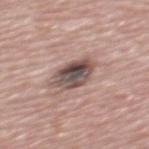Part of a total-body skin-imaging series; this lesion was reviewed on a skin check and was not flagged for biopsy.
The recorded lesion diameter is about 4.5 mm.
From the mid back.
A male subject aged 68–72.
Automated image analysis of the tile measured a lesion area of about 10 mm² and an outline eccentricity of about 0.65 (0 = round, 1 = elongated). It also reported a border-irregularity rating of about 2/10, a color-variation rating of about 10/10, and peripheral color asymmetry of about 3.5. It also reported a classifier nevus-likeness of about 10/100 and a detector confidence of about 100 out of 100 that the crop contains a lesion.
Imaged with white-light lighting.
A 15 mm close-up extracted from a 3D total-body photography capture.